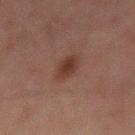lesion size: about 3 mm
lighting: cross-polarized illumination
acquisition: ~15 mm tile from a whole-body skin photo
location: the mid back
patient: male, approximately 60 years of age